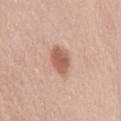Q: Is there a histopathology result?
A: imaged on a skin check; not biopsied
Q: What kind of image is this?
A: total-body-photography crop, ~15 mm field of view
Q: Where on the body is the lesion?
A: the mid back
Q: Lesion size?
A: ≈4 mm
Q: What lighting was used for the tile?
A: white-light illumination
Q: Who is the patient?
A: female, aged 48 to 52